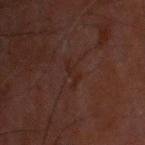Clinical impression:
The lesion was tiled from a total-body skin photograph and was not biopsied.
Acquisition and patient details:
A male patient aged 48 to 52. Located on the head or neck. The lesion's longest dimension is about 2.5 mm. An algorithmic analysis of the crop reported a footprint of about 3 mm². And it measured a mean CIELAB color near L≈17 a*≈15 b*≈17, a lesion–skin lightness drop of about 3, and a lesion-to-skin contrast of about 5 (normalized; higher = more distinct). This is a cross-polarized tile. This image is a 15 mm lesion crop taken from a total-body photograph.